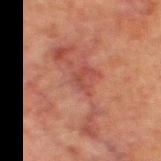Q: Was this lesion biopsied?
A: no biopsy performed (imaged during a skin exam)
Q: What is the imaging modality?
A: ~15 mm crop, total-body skin-cancer survey
Q: How was the tile lit?
A: cross-polarized illumination
Q: Patient demographics?
A: male, roughly 80 years of age
Q: What did automated image analysis measure?
A: an area of roughly 22 mm² and a shape eccentricity near 0.95; an average lesion color of about L≈39 a*≈22 b*≈23 (CIELAB), roughly 6 lightness units darker than nearby skin, and a normalized border contrast of about 5.5; border irregularity of about 10 on a 0–10 scale
Q: How large is the lesion?
A: ~10.5 mm (longest diameter)
Q: What is the anatomic site?
A: the left thigh